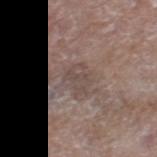Assessment: Part of a total-body skin-imaging series; this lesion was reviewed on a skin check and was not flagged for biopsy.